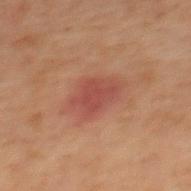The lesion is on the mid back.
Imaged with cross-polarized lighting.
A male patient roughly 70 years of age.
Cropped from a whole-body photographic skin survey; the tile spans about 15 mm.
About 3.5 mm across.